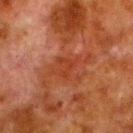workup — no biopsy performed (imaged during a skin exam)
anatomic site — the left lower leg
acquisition — total-body-photography crop, ~15 mm field of view
patient — male, aged 78 to 82